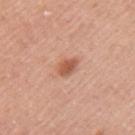workup = no biopsy performed (imaged during a skin exam)
patient = female, aged around 40
body site = the arm
acquisition = ~15 mm tile from a whole-body skin photo